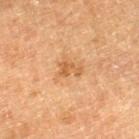Acquisition and patient details: Automated tile analysis of the lesion measured an area of roughly 3 mm² and two-axis asymmetry of about 0.55. And it measured about 8 CIELAB-L* units darker than the surrounding skin and a normalized lesion–skin contrast near 6.5. The lesion is located on the left thigh. A 15 mm crop from a total-body photograph taken for skin-cancer surveillance. A male patient, approximately 75 years of age. Measured at roughly 2.5 mm in maximum diameter.